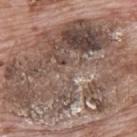The lesion was tiled from a total-body skin photograph and was not biopsied. About 17.5 mm across. Located on the upper back. Automated image analysis of the tile measured an average lesion color of about L≈50 a*≈15 b*≈23 (CIELAB), a lesion–skin lightness drop of about 11, and a normalized lesion–skin contrast near 8. The analysis additionally found a classifier nevus-likeness of about 0/100. A region of skin cropped from a whole-body photographic capture, roughly 15 mm wide. A male patient about 70 years old.